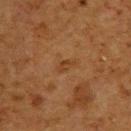Recorded during total-body skin imaging; not selected for excision or biopsy.
About 3 mm across.
A region of skin cropped from a whole-body photographic capture, roughly 15 mm wide.
The lesion is on the back.
The subject is a male approximately 60 years of age.
Captured under cross-polarized illumination.